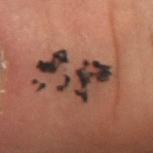{
  "lesion_size": {
    "long_diameter_mm_approx": 7.5
  },
  "image": {
    "source": "total-body photography crop",
    "field_of_view_mm": 15
  },
  "patient": {
    "sex": "male",
    "age_approx": 40
  },
  "site": "left forearm",
  "lighting": "cross-polarized"
}